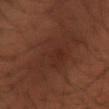Q: Was a biopsy performed?
A: total-body-photography surveillance lesion; no biopsy
Q: Patient demographics?
A: male, approximately 50 years of age
Q: Lesion location?
A: the arm
Q: What is the imaging modality?
A: 15 mm crop, total-body photography
Q: How was the tile lit?
A: cross-polarized illumination
Q: What is the lesion's diameter?
A: about 8.5 mm
Q: What did automated image analysis measure?
A: a footprint of about 29 mm² and a shape-asymmetry score of about 0.25 (0 = symmetric); an automated nevus-likeness rating near 15 out of 100 and a lesion-detection confidence of about 95/100